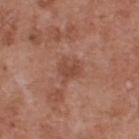Clinical impression:
This lesion was catalogued during total-body skin photography and was not selected for biopsy.
Background:
A male patient in their mid-50s. The tile uses white-light illumination. The total-body-photography lesion software estimated a border-irregularity rating of about 5/10, internal color variation of about 1.5 on a 0–10 scale, and a peripheral color-asymmetry measure near 0.5. And it measured a classifier nevus-likeness of about 0/100 and a detector confidence of about 100 out of 100 that the crop contains a lesion. This image is a 15 mm lesion crop taken from a total-body photograph. Approximately 3 mm at its widest. The lesion is located on the upper back.